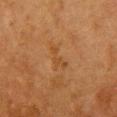Notes:
- patient: female, in their 50s
- body site: the chest
- lighting: cross-polarized
- image: ~15 mm tile from a whole-body skin photo
- size: ~3.5 mm (longest diameter)